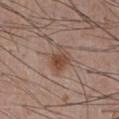Imaged during a routine full-body skin examination; the lesion was not biopsied and no histopathology is available. Longest diameter approximately 3 mm. The lesion is on the chest. The patient is a male approximately 55 years of age. Cropped from a total-body skin-imaging series; the visible field is about 15 mm. The total-body-photography lesion software estimated a detector confidence of about 100 out of 100 that the crop contains a lesion. Imaged with white-light lighting.